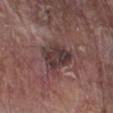follow-up — total-body-photography surveillance lesion; no biopsy
image source — ~15 mm tile from a whole-body skin photo
tile lighting — white-light
subject — male, aged approximately 80
diameter — ~4 mm (longest diameter)
anatomic site — the leg
automated lesion analysis — border irregularity of about 3 on a 0–10 scale, a within-lesion color-variation index near 4/10, and a peripheral color-asymmetry measure near 1.5